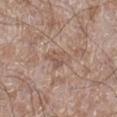biopsy_status: not biopsied; imaged during a skin examination
lighting: white-light
automated_metrics:
  border_irregularity_0_10: 4.0
  color_variation_0_10: 2.5
lesion_size:
  long_diameter_mm_approx: 3.5
patient:
  sex: male
  age_approx: 60
image:
  source: total-body photography crop
  field_of_view_mm: 15
site: right lower leg